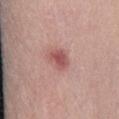<record>
  <automated_metrics>
    <cielab_L>53</cielab_L>
    <cielab_a>27</cielab_a>
    <cielab_b>23</cielab_b>
    <vs_skin_darker_L>11.0</vs_skin_darker_L>
    <nevus_likeness_0_100>20</nevus_likeness_0_100>
    <lesion_detection_confidence_0_100>100</lesion_detection_confidence_0_100>
  </automated_metrics>
  <patient>
    <sex>male</sex>
    <age_approx>30</age_approx>
  </patient>
  <lesion_size>
    <long_diameter_mm_approx>2.5</long_diameter_mm_approx>
  </lesion_size>
  <lighting>white-light</lighting>
  <image>
    <source>total-body photography crop</source>
    <field_of_view_mm>15</field_of_view_mm>
  </image>
</record>